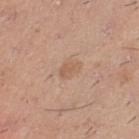{
  "biopsy_status": "not biopsied; imaged during a skin examination",
  "lighting": "white-light",
  "lesion_size": {
    "long_diameter_mm_approx": 2.5
  },
  "site": "left thigh",
  "image": {
    "source": "total-body photography crop",
    "field_of_view_mm": 15
  },
  "automated_metrics": {
    "area_mm2_approx": 4.0,
    "eccentricity": 0.75,
    "shape_asymmetry": 0.3,
    "border_irregularity_0_10": 3.0,
    "color_variation_0_10": 1.5,
    "peripheral_color_asymmetry": 0.5,
    "nevus_likeness_0_100": 15,
    "lesion_detection_confidence_0_100": 100
  },
  "patient": {
    "sex": "male",
    "age_approx": 40
  }
}